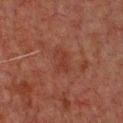A male patient in their 60s.
Located on the front of the torso.
A 15 mm crop from a total-body photograph taken for skin-cancer surveillance.